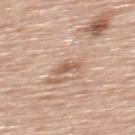follow-up = no biopsy performed (imaged during a skin exam) | subject = male, aged approximately 60 | imaging modality = 15 mm crop, total-body photography | lesion size = ~3 mm (longest diameter) | automated lesion analysis = a footprint of about 3 mm² and an eccentricity of roughly 0.9; lesion-presence confidence of about 95/100 | site = the back.